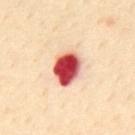Assessment: The lesion was tiled from a total-body skin photograph and was not biopsied. Background: Located on the mid back. A male patient aged 53 to 57. An algorithmic analysis of the crop reported a shape eccentricity near 0.6. The analysis additionally found a lesion–skin lightness drop of about 29 and a normalized border contrast of about 16. A lesion tile, about 15 mm wide, cut from a 3D total-body photograph. The tile uses cross-polarized illumination.